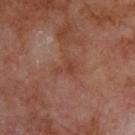Notes:
* biopsy status — imaged on a skin check; not biopsied
* subject — male, aged approximately 70
* anatomic site — the upper back
* tile lighting — cross-polarized
* imaging modality — ~15 mm tile from a whole-body skin photo
* automated metrics — an average lesion color of about L≈40 a*≈23 b*≈28 (CIELAB), a lesion–skin lightness drop of about 6, and a lesion-to-skin contrast of about 5.5 (normalized; higher = more distinct); a border-irregularity rating of about 5.5/10, internal color variation of about 1 on a 0–10 scale, and radial color variation of about 0; a lesion-detection confidence of about 100/100